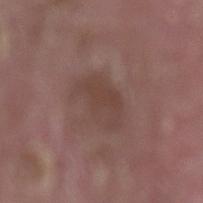Impression:
Recorded during total-body skin imaging; not selected for excision or biopsy.
Image and clinical context:
On the lower back. The subject is a male roughly 40 years of age. Cropped from a whole-body photographic skin survey; the tile spans about 15 mm. Measured at roughly 5 mm in maximum diameter. Captured under white-light illumination. Automated image analysis of the tile measured an automated nevus-likeness rating near 0 out of 100 and a lesion-detection confidence of about 100/100.